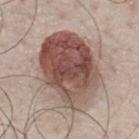workup: imaged on a skin check; not biopsied | body site: the chest | image source: ~15 mm tile from a whole-body skin photo | patient: male, aged 48 to 52 | tile lighting: white-light illumination | diameter: ≈9 mm.